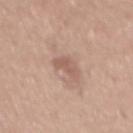biopsy status: no biopsy performed (imaged during a skin exam) | lesion size: ≈2.5 mm | patient: male, aged 28 to 32 | image: total-body-photography crop, ~15 mm field of view | location: the mid back | lighting: white-light.